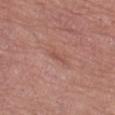Clinical impression: Imaged during a routine full-body skin examination; the lesion was not biopsied and no histopathology is available. Image and clinical context: The subject is a female aged approximately 65. Measured at roughly 2.5 mm in maximum diameter. The tile uses white-light illumination. From the left thigh. A lesion tile, about 15 mm wide, cut from a 3D total-body photograph.